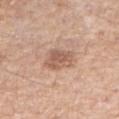This is a white-light tile.
Cropped from a total-body skin-imaging series; the visible field is about 15 mm.
A male subject, aged around 60.
Automated image analysis of the tile measured a mean CIELAB color near L≈58 a*≈21 b*≈29, a lesion–skin lightness drop of about 10, and a lesion-to-skin contrast of about 7 (normalized; higher = more distinct). The analysis additionally found a nevus-likeness score of about 10/100.
The lesion is on the right forearm.
About 3.5 mm across.